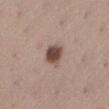follow-up=no biopsy performed (imaged during a skin exam); subject=female, aged 43 to 47; body site=the left lower leg; tile lighting=white-light illumination; size=about 2.5 mm; image source=~15 mm crop, total-body skin-cancer survey.